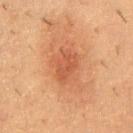Impression:
Imaged during a routine full-body skin examination; the lesion was not biopsied and no histopathology is available.
Context:
From the upper back. A lesion tile, about 15 mm wide, cut from a 3D total-body photograph. This is a cross-polarized tile. An algorithmic analysis of the crop reported an eccentricity of roughly 0.8 and a shape-asymmetry score of about 0.2 (0 = symmetric). And it measured a lesion color around L≈47 a*≈25 b*≈34 in CIELAB and about 7 CIELAB-L* units darker than the surrounding skin. The patient is a male in their mid-50s. About 3.5 mm across.